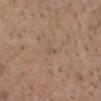notes = catalogued during a skin exam; not biopsied | patient = male, aged 53–57 | image source = 15 mm crop, total-body photography | size = about 1 mm | tile lighting = white-light | site = the head or neck.